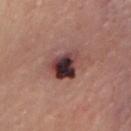| key | value |
|---|---|
| follow-up | no biopsy performed (imaged during a skin exam) |
| image | ~15 mm tile from a whole-body skin photo |
| anatomic site | the head or neck |
| patient | male, aged 78–82 |
| tile lighting | white-light |
| diameter | ≈4 mm |